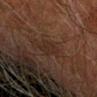biopsy status — imaged on a skin check; not biopsied
size — ≈3 mm
image — ~15 mm crop, total-body skin-cancer survey
anatomic site — the right forearm
subject — male, in their mid- to late 70s